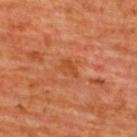Imaged during a routine full-body skin examination; the lesion was not biopsied and no histopathology is available.
A male patient in their mid- to late 60s.
From the upper back.
A roughly 15 mm field-of-view crop from a total-body skin photograph.
This is a cross-polarized tile.
Measured at roughly 3 mm in maximum diameter.